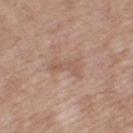This lesion was catalogued during total-body skin photography and was not selected for biopsy.
A female patient, aged 53–57.
On the right thigh.
This image is a 15 mm lesion crop taken from a total-body photograph.